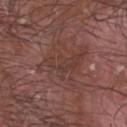| field | value |
|---|---|
| biopsy status | catalogued during a skin exam; not biopsied |
| subject | male, approximately 45 years of age |
| illumination | white-light illumination |
| automated lesion analysis | a footprint of about 10 mm², an eccentricity of roughly 0.9, and two-axis asymmetry of about 0.55; a border-irregularity rating of about 9/10, a within-lesion color-variation index near 2.5/10, and peripheral color asymmetry of about 0.5 |
| site | the head or neck |
| acquisition | total-body-photography crop, ~15 mm field of view |